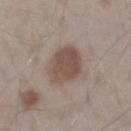Imaged during a routine full-body skin examination; the lesion was not biopsied and no histopathology is available. A 15 mm crop from a total-body photograph taken for skin-cancer surveillance. The recorded lesion diameter is about 4.5 mm. The lesion is on the right upper arm. The patient is a male about 55 years old. Captured under white-light illumination.